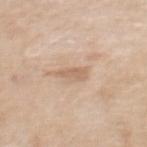Q: Was this lesion biopsied?
A: no biopsy performed (imaged during a skin exam)
Q: How large is the lesion?
A: ~2.5 mm (longest diameter)
Q: What did automated image analysis measure?
A: a mean CIELAB color near L≈63 a*≈17 b*≈31, about 9 CIELAB-L* units darker than the surrounding skin, and a normalized border contrast of about 6; peripheral color asymmetry of about 0; a nevus-likeness score of about 0/100
Q: Who is the patient?
A: female, approximately 60 years of age
Q: What is the anatomic site?
A: the upper back
Q: What kind of image is this?
A: total-body-photography crop, ~15 mm field of view
Q: What lighting was used for the tile?
A: white-light